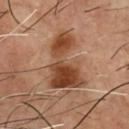Assessment:
The lesion was tiled from a total-body skin photograph and was not biopsied.
Image and clinical context:
The total-body-photography lesion software estimated an average lesion color of about L≈43 a*≈22 b*≈32 (CIELAB) and a lesion–skin lightness drop of about 13. A close-up tile cropped from a whole-body skin photograph, about 15 mm across. On the chest. A male patient about 55 years old. Captured under cross-polarized illumination.